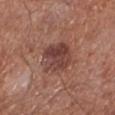Q: Was this lesion biopsied?
A: imaged on a skin check; not biopsied
Q: What are the patient's age and sex?
A: male, about 70 years old
Q: Automated lesion metrics?
A: a footprint of about 12 mm², an outline eccentricity of about 0.55 (0 = round, 1 = elongated), and two-axis asymmetry of about 0.15; a mean CIELAB color near L≈41 a*≈22 b*≈23, about 10 CIELAB-L* units darker than the surrounding skin, and a lesion-to-skin contrast of about 8.5 (normalized; higher = more distinct); a border-irregularity rating of about 2/10, internal color variation of about 5.5 on a 0–10 scale, and radial color variation of about 2
Q: What is the anatomic site?
A: the left lower leg
Q: How was the tile lit?
A: white-light
Q: What kind of image is this?
A: ~15 mm crop, total-body skin-cancer survey